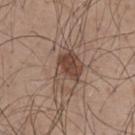Q: What did automated image analysis measure?
A: a lesion color around L≈45 a*≈17 b*≈25 in CIELAB and a normalized border contrast of about 8.5; a border-irregularity index near 6/10, internal color variation of about 3.5 on a 0–10 scale, and radial color variation of about 1
Q: What kind of image is this?
A: ~15 mm crop, total-body skin-cancer survey
Q: How was the tile lit?
A: white-light illumination
Q: Patient demographics?
A: male, aged 48 to 52
Q: Where on the body is the lesion?
A: the upper back